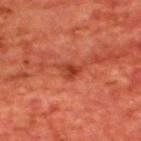workup: catalogued during a skin exam; not biopsied | patient: male, approximately 65 years of age | image: 15 mm crop, total-body photography | image-analysis metrics: an automated nevus-likeness rating near 10 out of 100 and a lesion-detection confidence of about 100/100 | site: the upper back | size: about 2.5 mm | lighting: cross-polarized.